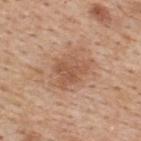The lesion was photographed on a routine skin check and not biopsied; there is no pathology result. The lesion-visualizer software estimated a footprint of about 10 mm². And it measured border irregularity of about 4 on a 0–10 scale and a color-variation rating of about 3.5/10. Located on the upper back. This is a white-light tile. A male subject aged 58–62. A region of skin cropped from a whole-body photographic capture, roughly 15 mm wide. The lesion's longest dimension is about 4.5 mm.